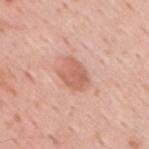Findings:
- tile lighting — white-light illumination
- image — ~15 mm tile from a whole-body skin photo
- automated metrics — an area of roughly 8 mm² and an outline eccentricity of about 0.65 (0 = round, 1 = elongated); a classifier nevus-likeness of about 10/100 and lesion-presence confidence of about 100/100
- anatomic site — the upper back
- subject — male, in their mid- to late 30s
- diameter — ~3.5 mm (longest diameter)